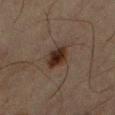Clinical impression: Recorded during total-body skin imaging; not selected for excision or biopsy. Clinical summary: The total-body-photography lesion software estimated a detector confidence of about 100 out of 100 that the crop contains a lesion. On the right upper arm. A 15 mm close-up extracted from a 3D total-body photography capture. The tile uses cross-polarized illumination. A male patient, in their mid-60s. About 3 mm across.